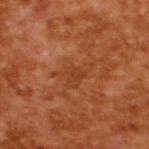This lesion was catalogued during total-body skin photography and was not selected for biopsy.
A lesion tile, about 15 mm wide, cut from a 3D total-body photograph.
A male subject in their mid- to late 60s.